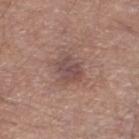{
  "image": {
    "source": "total-body photography crop",
    "field_of_view_mm": 15
  },
  "site": "left lower leg",
  "patient": {
    "sex": "male",
    "age_approx": 65
  }
}